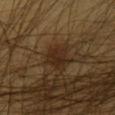follow-up — total-body-photography surveillance lesion; no biopsy | patient — male, aged 63–67 | illumination — cross-polarized | TBP lesion metrics — a footprint of about 6 mm², an eccentricity of roughly 0.65, and a shape-asymmetry score of about 0.25 (0 = symmetric); a mean CIELAB color near L≈26 a*≈16 b*≈27 and a lesion–skin lightness drop of about 7; a color-variation rating of about 3/10 and radial color variation of about 1; a lesion-detection confidence of about 95/100 | body site — the chest | image — ~15 mm crop, total-body skin-cancer survey.